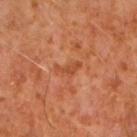Assessment:
The lesion was photographed on a routine skin check and not biopsied; there is no pathology result.
Image and clinical context:
A male subject aged 58–62. Cropped from a total-body skin-imaging series; the visible field is about 15 mm. From the arm. Captured under cross-polarized illumination. Automated image analysis of the tile measured border irregularity of about 6 on a 0–10 scale, internal color variation of about 1 on a 0–10 scale, and a peripheral color-asymmetry measure near 0.5. It also reported an automated nevus-likeness rating near 0 out of 100. Measured at roughly 3 mm in maximum diameter.